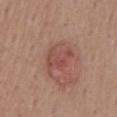| key | value |
|---|---|
| biopsy status | total-body-photography surveillance lesion; no biopsy |